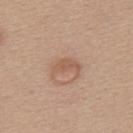biopsy status: imaged on a skin check; not biopsied
imaging modality: ~15 mm crop, total-body skin-cancer survey
diameter: ≈3.5 mm
patient: female, roughly 40 years of age
image-analysis metrics: a footprint of about 4 mm², a shape eccentricity near 0.85, and a shape-asymmetry score of about 0.45 (0 = symmetric); a lesion color around L≈56 a*≈20 b*≈30 in CIELAB, a lesion–skin lightness drop of about 8, and a lesion-to-skin contrast of about 6 (normalized; higher = more distinct)
lighting: white-light
anatomic site: the upper back